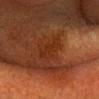{"biopsy_status": "not biopsied; imaged during a skin examination", "lighting": "cross-polarized", "site": "head or neck", "lesion_size": {"long_diameter_mm_approx": 4.0}, "patient": {"sex": "female", "age_approx": 40}, "image": {"source": "total-body photography crop", "field_of_view_mm": 15}}